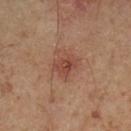Part of a total-body skin-imaging series; this lesion was reviewed on a skin check and was not flagged for biopsy. Imaged with cross-polarized lighting. A male subject in their mid- to late 50s. On the leg. Longest diameter approximately 3 mm. A 15 mm crop from a total-body photograph taken for skin-cancer surveillance. Automated tile analysis of the lesion measured an area of roughly 5.5 mm² and two-axis asymmetry of about 0.35. The analysis additionally found an average lesion color of about L≈43 a*≈23 b*≈27 (CIELAB), a lesion–skin lightness drop of about 8, and a normalized border contrast of about 6.5. And it measured a peripheral color-asymmetry measure near 1.5. And it measured a classifier nevus-likeness of about 5/100 and a detector confidence of about 100 out of 100 that the crop contains a lesion.